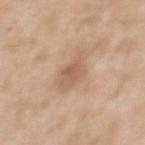Notes:
* biopsy status: imaged on a skin check; not biopsied
* location: the back
* imaging modality: 15 mm crop, total-body photography
* patient: female, aged around 40
* tile lighting: white-light illumination
* size: about 4 mm